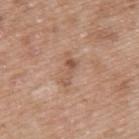Imaged during a routine full-body skin examination; the lesion was not biopsied and no histopathology is available. A male patient, aged 48–52. The lesion is on the upper back. A 15 mm close-up tile from a total-body photography series done for melanoma screening. The tile uses white-light illumination.